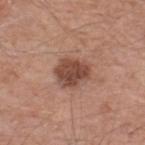The lesion was tiled from a total-body skin photograph and was not biopsied. About 4 mm across. This is a white-light tile. A region of skin cropped from a whole-body photographic capture, roughly 15 mm wide. From the upper back. The subject is a male aged around 55. The total-body-photography lesion software estimated an area of roughly 11 mm², an eccentricity of roughly 0.55, and two-axis asymmetry of about 0.2. And it measured border irregularity of about 2 on a 0–10 scale and peripheral color asymmetry of about 1. The software also gave an automated nevus-likeness rating near 60 out of 100 and lesion-presence confidence of about 100/100.